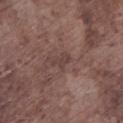Q: Was this lesion biopsied?
A: no biopsy performed (imaged during a skin exam)
Q: Automated lesion metrics?
A: an area of roughly 3 mm²; a peripheral color-asymmetry measure near 0; an automated nevus-likeness rating near 0 out of 100 and a detector confidence of about 70 out of 100 that the crop contains a lesion
Q: How large is the lesion?
A: about 2.5 mm
Q: What is the imaging modality?
A: 15 mm crop, total-body photography
Q: Who is the patient?
A: male, in their mid-70s
Q: Lesion location?
A: the left lower leg